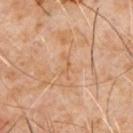  biopsy_status: not biopsied; imaged during a skin examination
  lesion_size:
    long_diameter_mm_approx: 2.5
  lighting: cross-polarized
  image:
    source: total-body photography crop
    field_of_view_mm: 15
  site: front of the torso
  patient:
    sex: male
    age_approx: 60